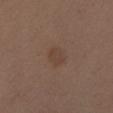Part of a total-body skin-imaging series; this lesion was reviewed on a skin check and was not flagged for biopsy. A female patient aged around 40. A region of skin cropped from a whole-body photographic capture, roughly 15 mm wide. The lesion is located on the left thigh. This is a white-light tile. The lesion's longest dimension is about 2.5 mm. Automated tile analysis of the lesion measured a footprint of about 4.5 mm² and a shape-asymmetry score of about 0.25 (0 = symmetric). It also reported a border-irregularity index near 2/10 and internal color variation of about 2 on a 0–10 scale. And it measured a nevus-likeness score of about 10/100 and lesion-presence confidence of about 100/100.